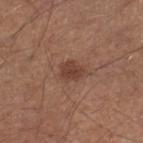follow-up = imaged on a skin check; not biopsied | illumination = white-light | acquisition = ~15 mm tile from a whole-body skin photo | subject = male, approximately 65 years of age | body site = the right lower leg.